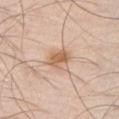Part of a total-body skin-imaging series; this lesion was reviewed on a skin check and was not flagged for biopsy.
Captured under white-light illumination.
A male subject roughly 30 years of age.
The recorded lesion diameter is about 3 mm.
Cropped from a total-body skin-imaging series; the visible field is about 15 mm.
The lesion is located on the front of the torso.